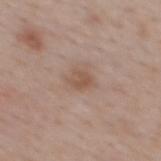{"biopsy_status": "not biopsied; imaged during a skin examination", "site": "mid back", "image": {"source": "total-body photography crop", "field_of_view_mm": 15}, "lighting": "white-light", "patient": {"sex": "male", "age_approx": 40}, "lesion_size": {"long_diameter_mm_approx": 2.5}}